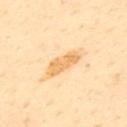follow-up: total-body-photography surveillance lesion; no biopsy
subject: male, roughly 55 years of age
image source: ~15 mm tile from a whole-body skin photo
lesion size: ~5 mm (longest diameter)
body site: the back
tile lighting: cross-polarized illumination
image-analysis metrics: a footprint of about 7.5 mm², a shape eccentricity near 0.9, and a shape-asymmetry score of about 0.25 (0 = symmetric); a border-irregularity index near 3/10, internal color variation of about 2.5 on a 0–10 scale, and radial color variation of about 1